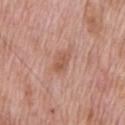{
  "biopsy_status": "not biopsied; imaged during a skin examination",
  "site": "chest",
  "lesion_size": {
    "long_diameter_mm_approx": 3.0
  },
  "patient": {
    "sex": "male",
    "age_approx": 70
  },
  "image": {
    "source": "total-body photography crop",
    "field_of_view_mm": 15
  }
}